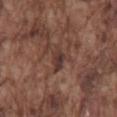A lesion tile, about 15 mm wide, cut from a 3D total-body photograph.
Automated image analysis of the tile measured an outline eccentricity of about 0.85 (0 = round, 1 = elongated) and a symmetry-axis asymmetry near 0.3. It also reported an average lesion color of about L≈36 a*≈18 b*≈22 (CIELAB), roughly 8 lightness units darker than nearby skin, and a lesion-to-skin contrast of about 7.5 (normalized; higher = more distinct). The analysis additionally found border irregularity of about 3.5 on a 0–10 scale, a within-lesion color-variation index near 3/10, and a peripheral color-asymmetry measure near 1.
Captured under white-light illumination.
From the mid back.
A male patient aged 73–77.
Approximately 3.5 mm at its widest.